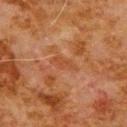notes = no biopsy performed (imaged during a skin exam).